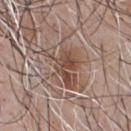No biopsy was performed on this lesion — it was imaged during a full skin examination and was not determined to be concerning. Measured at roughly 6.5 mm in maximum diameter. The subject is a male roughly 45 years of age. Captured under white-light illumination. Automated image analysis of the tile measured a footprint of about 17 mm², an outline eccentricity of about 0.75 (0 = round, 1 = elongated), and two-axis asymmetry of about 0.5. It also reported an automated nevus-likeness rating near 70 out of 100 and lesion-presence confidence of about 80/100. Cropped from a whole-body photographic skin survey; the tile spans about 15 mm. The lesion is on the chest.